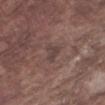This lesion was catalogued during total-body skin photography and was not selected for biopsy. The tile uses white-light illumination. An algorithmic analysis of the crop reported a border-irregularity index near 5/10 and peripheral color asymmetry of about 0. From the arm. A roughly 15 mm field-of-view crop from a total-body skin photograph. The recorded lesion diameter is about 3 mm. The patient is a male in their mid-70s.